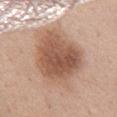{"biopsy_status": "not biopsied; imaged during a skin examination", "site": "chest", "lighting": "white-light", "lesion_size": {"long_diameter_mm_approx": 8.5}, "image": {"source": "total-body photography crop", "field_of_view_mm": 15}, "automated_metrics": {"border_irregularity_0_10": 2.5, "color_variation_0_10": 5.0, "peripheral_color_asymmetry": 1.5, "nevus_likeness_0_100": 95, "lesion_detection_confidence_0_100": 100}, "patient": {"sex": "female", "age_approx": 65}}